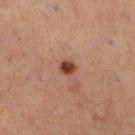notes = imaged on a skin check; not biopsied | lesion size = ≈2 mm | image source = 15 mm crop, total-body photography | body site = the right lower leg | subject = female, aged 53 to 57 | TBP lesion metrics = a lesion color around L≈40 a*≈22 b*≈29 in CIELAB, roughly 13 lightness units darker than nearby skin, and a normalized border contrast of about 11; a classifier nevus-likeness of about 95/100 | lighting = cross-polarized.